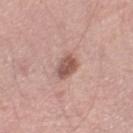lesion size — about 2.5 mm | patient — male, approximately 50 years of age | image source — ~15 mm crop, total-body skin-cancer survey | site — the right thigh | automated metrics — a footprint of about 5 mm², an eccentricity of roughly 0.55, and a shape-asymmetry score of about 0.15 (0 = symmetric); a border-irregularity rating of about 1.5/10 and internal color variation of about 3 on a 0–10 scale; an automated nevus-likeness rating near 60 out of 100 and a lesion-detection confidence of about 100/100 | tile lighting — white-light illumination.